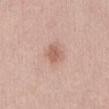{
  "biopsy_status": "not biopsied; imaged during a skin examination",
  "patient": {
    "sex": "female",
    "age_approx": 45
  },
  "lesion_size": {
    "long_diameter_mm_approx": 2.5
  },
  "site": "abdomen",
  "image": {
    "source": "total-body photography crop",
    "field_of_view_mm": 15
  }
}